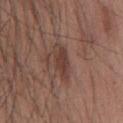The lesion was photographed on a routine skin check and not biopsied; there is no pathology result.